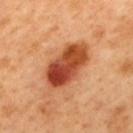Impression: This lesion was catalogued during total-body skin photography and was not selected for biopsy. Clinical summary: Automated image analysis of the tile measured a lesion area of about 19 mm². It also reported a lesion–skin lightness drop of about 17 and a normalized border contrast of about 11.5. The software also gave a border-irregularity index near 1.5/10 and internal color variation of about 9 on a 0–10 scale. Located on the mid back. Approximately 6.5 mm at its widest. A 15 mm close-up extracted from a 3D total-body photography capture. A male subject, aged 48 to 52. Captured under cross-polarized illumination.